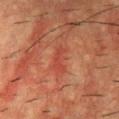biopsy_status: not biopsied; imaged during a skin examination
site: upper back
image:
  source: total-body photography crop
  field_of_view_mm: 15
patient:
  sex: male
  age_approx: 55
lighting: cross-polarized
lesion_size:
  long_diameter_mm_approx: 3.5